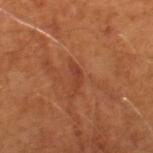  biopsy_status: not biopsied; imaged during a skin examination
  image:
    source: total-body photography crop
    field_of_view_mm: 15
  automated_metrics:
    cielab_L: 40
    cielab_a: 27
    cielab_b: 33
    vs_skin_darker_L: 7.0
    vs_skin_contrast_norm: 5.5
  patient:
    sex: male
    age_approx: 65
  lighting: cross-polarized